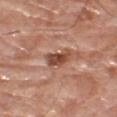This lesion was catalogued during total-body skin photography and was not selected for biopsy.
This is a white-light tile.
This image is a 15 mm lesion crop taken from a total-body photograph.
A male subject, roughly 80 years of age.
The lesion is on the leg.
Approximately 4 mm at its widest.